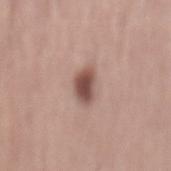Assessment: This lesion was catalogued during total-body skin photography and was not selected for biopsy.